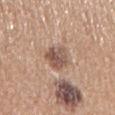workup: imaged on a skin check; not biopsied
acquisition: ~15 mm tile from a whole-body skin photo
patient: female, aged 63–67
location: the right upper arm